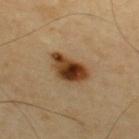The lesion was tiled from a total-body skin photograph and was not biopsied.
The lesion is located on the upper back.
Cropped from a total-body skin-imaging series; the visible field is about 15 mm.
The patient is a male about 65 years old.